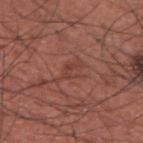{"biopsy_status": "not biopsied; imaged during a skin examination", "image": {"source": "total-body photography crop", "field_of_view_mm": 15}, "site": "upper back", "lesion_size": {"long_diameter_mm_approx": 3.5}, "patient": {"sex": "male", "age_approx": 35}, "lighting": "white-light", "automated_metrics": {"area_mm2_approx": 4.5, "eccentricity": 0.85, "shape_asymmetry": 0.4, "color_variation_0_10": 2.0, "peripheral_color_asymmetry": 0.5, "nevus_likeness_0_100": 0}}